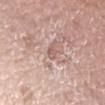Q: Is there a histopathology result?
A: catalogued during a skin exam; not biopsied
Q: What kind of image is this?
A: ~15 mm crop, total-body skin-cancer survey
Q: What are the patient's age and sex?
A: female, aged 48 to 52
Q: Lesion location?
A: the right forearm